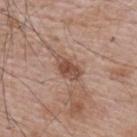biopsy_status: not biopsied; imaged during a skin examination
site: back
patient:
  sex: male
  age_approx: 65
lesion_size:
  long_diameter_mm_approx: 4.0
image:
  source: total-body photography crop
  field_of_view_mm: 15
lighting: white-light